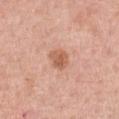workup: total-body-photography surveillance lesion; no biopsy
automated metrics: a lesion area of about 5.5 mm², a shape eccentricity near 0.4, and two-axis asymmetry of about 0.25; a border-irregularity rating of about 2/10 and peripheral color asymmetry of about 1.5; a nevus-likeness score of about 50/100 and lesion-presence confidence of about 100/100
subject: male, in their 60s
lesion size: about 2.5 mm
site: the front of the torso
imaging modality: ~15 mm crop, total-body skin-cancer survey